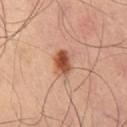The lesion was tiled from a total-body skin photograph and was not biopsied. The lesion is on the leg. The recorded lesion diameter is about 3.5 mm. A roughly 15 mm field-of-view crop from a total-body skin photograph.